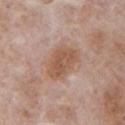notes: no biopsy performed (imaged during a skin exam) | subject: male, aged approximately 70 | location: the front of the torso | image source: ~15 mm tile from a whole-body skin photo.